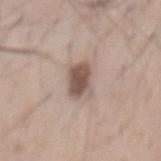No biopsy was performed on this lesion — it was imaged during a full skin examination and was not determined to be concerning. Located on the mid back. A male patient, aged 48 to 52. A lesion tile, about 15 mm wide, cut from a 3D total-body photograph.